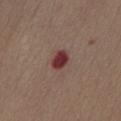This lesion was catalogued during total-body skin photography and was not selected for biopsy.
The lesion-visualizer software estimated a footprint of about 5 mm² and a shape-asymmetry score of about 0.2 (0 = symmetric). And it measured a mean CIELAB color near L≈36 a*≈25 b*≈20, roughly 14 lightness units darker than nearby skin, and a normalized lesion–skin contrast near 11.5.
A male patient, approximately 75 years of age.
A region of skin cropped from a whole-body photographic capture, roughly 15 mm wide.
Located on the front of the torso.
About 3 mm across.